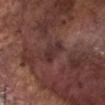Findings:
- follow-up · no biopsy performed (imaged during a skin exam)
- patient · male, approximately 75 years of age
- tile lighting · white-light
- automated metrics · a lesion area of about 5 mm² and a shape eccentricity near 0.9; a nevus-likeness score of about 0/100 and lesion-presence confidence of about 85/100
- anatomic site · the head or neck
- diameter · ≈3.5 mm
- imaging modality · ~15 mm crop, total-body skin-cancer survey